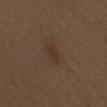Case summary:
- image source · total-body-photography crop, ~15 mm field of view
- subject · male, in their 70s
- anatomic site · the mid back